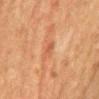{
  "site": "back",
  "lighting": "cross-polarized",
  "patient": {
    "sex": "female",
    "age_approx": 60
  },
  "image": {
    "source": "total-body photography crop",
    "field_of_view_mm": 15
  },
  "lesion_size": {
    "long_diameter_mm_approx": 2.5
  }
}